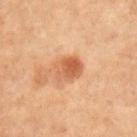Part of a total-body skin-imaging series; this lesion was reviewed on a skin check and was not flagged for biopsy.
A male subject aged 68 to 72.
Imaged with cross-polarized lighting.
Longest diameter approximately 3.5 mm.
A 15 mm crop from a total-body photograph taken for skin-cancer surveillance.
On the right upper arm.